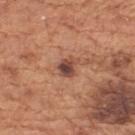This lesion was catalogued during total-body skin photography and was not selected for biopsy. The tile uses white-light illumination. A 15 mm crop from a total-body photograph taken for skin-cancer surveillance. The lesion is on the mid back. Measured at roughly 2.5 mm in maximum diameter. A male subject aged 63 to 67.